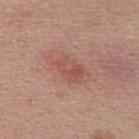Q: Was a biopsy performed?
A: imaged on a skin check; not biopsied
Q: Patient demographics?
A: female, aged 43 to 47
Q: What is the imaging modality?
A: 15 mm crop, total-body photography
Q: Automated lesion metrics?
A: a lesion-detection confidence of about 100/100
Q: Lesion location?
A: the upper back